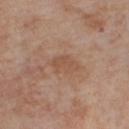workup=total-body-photography surveillance lesion; no biopsy
subject=female, in their mid- to late 50s
anatomic site=the right thigh
acquisition=~15 mm crop, total-body skin-cancer survey